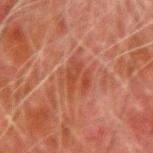The lesion was tiled from a total-body skin photograph and was not biopsied.
The lesion is on the arm.
The lesion-visualizer software estimated border irregularity of about 4 on a 0–10 scale, internal color variation of about 3.5 on a 0–10 scale, and a peripheral color-asymmetry measure near 1.
Imaged with cross-polarized lighting.
A 15 mm close-up tile from a total-body photography series done for melanoma screening.
Longest diameter approximately 5 mm.
A male subject, aged 78 to 82.